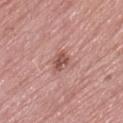Q: Was a biopsy performed?
A: no biopsy performed (imaged during a skin exam)
Q: Lesion location?
A: the left thigh
Q: Patient demographics?
A: female, about 70 years old
Q: Automated lesion metrics?
A: an area of roughly 4.5 mm² and a symmetry-axis asymmetry near 0.25; a lesion color around L≈53 a*≈24 b*≈24 in CIELAB, about 11 CIELAB-L* units darker than the surrounding skin, and a lesion-to-skin contrast of about 7.5 (normalized; higher = more distinct); border irregularity of about 2.5 on a 0–10 scale, a within-lesion color-variation index near 3/10, and radial color variation of about 1; an automated nevus-likeness rating near 30 out of 100
Q: What is the imaging modality?
A: ~15 mm tile from a whole-body skin photo